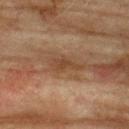The lesion was photographed on a routine skin check and not biopsied; there is no pathology result.
This image is a 15 mm lesion crop taken from a total-body photograph.
The lesion is located on the upper back.
A male patient, roughly 75 years of age.